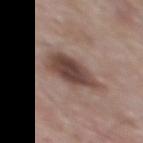Assessment:
Imaged during a routine full-body skin examination; the lesion was not biopsied and no histopathology is available.
Acquisition and patient details:
A male subject aged 63 to 67. A close-up tile cropped from a whole-body skin photograph, about 15 mm across. The lesion is located on the upper back.